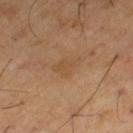Q: Was this lesion biopsied?
A: imaged on a skin check; not biopsied
Q: Lesion location?
A: the left thigh
Q: How was the tile lit?
A: cross-polarized illumination
Q: How was this image acquired?
A: ~15 mm crop, total-body skin-cancer survey
Q: Patient demographics?
A: male, in their mid- to late 60s
Q: What is the lesion's diameter?
A: ~3.5 mm (longest diameter)